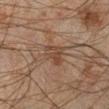Recorded during total-body skin imaging; not selected for excision or biopsy.
A region of skin cropped from a whole-body photographic capture, roughly 15 mm wide.
This is a cross-polarized tile.
The subject is a male about 45 years old.
Located on the leg.
Automated tile analysis of the lesion measured a shape eccentricity near 0.8 and a symmetry-axis asymmetry near 0.35. The software also gave a border-irregularity index near 3.5/10, a color-variation rating of about 3.5/10, and peripheral color asymmetry of about 1.5. And it measured an automated nevus-likeness rating near 0 out of 100 and a detector confidence of about 100 out of 100 that the crop contains a lesion.